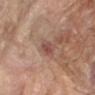Q: What did automated image analysis measure?
A: an average lesion color of about L≈45 a*≈21 b*≈23 (CIELAB) and a normalized lesion–skin contrast near 7; a border-irregularity rating of about 2/10 and peripheral color asymmetry of about 0.5; a lesion-detection confidence of about 100/100
Q: Who is the patient?
A: male, aged 63 to 67
Q: Lesion location?
A: the right forearm
Q: What is the imaging modality?
A: ~15 mm tile from a whole-body skin photo
Q: What is the lesion's diameter?
A: about 2.5 mm